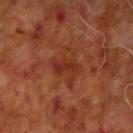Q: Is there a histopathology result?
A: no biopsy performed (imaged during a skin exam)
Q: Where on the body is the lesion?
A: the right upper arm
Q: What is the imaging modality?
A: total-body-photography crop, ~15 mm field of view
Q: What did automated image analysis measure?
A: a lesion area of about 5 mm², an outline eccentricity of about 0.85 (0 = round, 1 = elongated), and a symmetry-axis asymmetry near 0.35; an average lesion color of about L≈26 a*≈24 b*≈27 (CIELAB), about 5 CIELAB-L* units darker than the surrounding skin, and a normalized lesion–skin contrast near 6
Q: What is the lesion's diameter?
A: about 3.5 mm
Q: Patient demographics?
A: male, about 70 years old